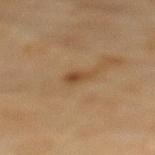Findings:
* biopsy status · catalogued during a skin exam; not biopsied
* image-analysis metrics · a footprint of about 3 mm², an outline eccentricity of about 0.85 (0 = round, 1 = elongated), and a symmetry-axis asymmetry near 0.4; a lesion color around L≈39 a*≈16 b*≈30 in CIELAB, about 8 CIELAB-L* units darker than the surrounding skin, and a normalized lesion–skin contrast near 7; a classifier nevus-likeness of about 70/100 and a detector confidence of about 100 out of 100 that the crop contains a lesion
* imaging modality · 15 mm crop, total-body photography
* subject · female, roughly 80 years of age
* illumination · cross-polarized illumination
* location · the mid back